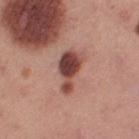Clinical impression:
The lesion was tiled from a total-body skin photograph and was not biopsied.
Image and clinical context:
Longest diameter approximately 5 mm. The lesion is on the right thigh. Imaged with white-light lighting. A female subject in their 40s. Cropped from a total-body skin-imaging series; the visible field is about 15 mm.